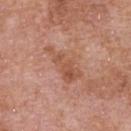  biopsy_status: not biopsied; imaged during a skin examination
  lighting: white-light
  patient:
    sex: male
    age_approx: 70
  site: chest
  lesion_size:
    long_diameter_mm_approx: 5.0
  image:
    source: total-body photography crop
    field_of_view_mm: 15
  automated_metrics:
    area_mm2_approx: 8.5
    eccentricity: 0.9
    shape_asymmetry: 0.5
    border_irregularity_0_10: 6.5
    peripheral_color_asymmetry: 1.0
    nevus_likeness_0_100: 0
    lesion_detection_confidence_0_100: 100